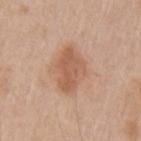Assessment:
No biopsy was performed on this lesion — it was imaged during a full skin examination and was not determined to be concerning.
Clinical summary:
Automated image analysis of the tile measured an outline eccentricity of about 0.8 (0 = round, 1 = elongated) and a symmetry-axis asymmetry near 0.2. The analysis additionally found a mean CIELAB color near L≈58 a*≈22 b*≈32, a lesion–skin lightness drop of about 10, and a normalized lesion–skin contrast near 6.5. The software also gave an automated nevus-likeness rating near 15 out of 100 and a lesion-detection confidence of about 100/100. A male subject aged around 60. A lesion tile, about 15 mm wide, cut from a 3D total-body photograph. This is a white-light tile. Approximately 5 mm at its widest. From the arm.